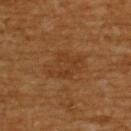Q: How large is the lesion?
A: ~5 mm (longest diameter)
Q: What kind of image is this?
A: 15 mm crop, total-body photography
Q: Lesion location?
A: the upper back
Q: Who is the patient?
A: male, about 60 years old
Q: What did automated image analysis measure?
A: an area of roughly 13 mm², a shape eccentricity near 0.75, and a shape-asymmetry score of about 0.25 (0 = symmetric); a lesion color around L≈35 a*≈19 b*≈32 in CIELAB, about 5 CIELAB-L* units darker than the surrounding skin, and a normalized lesion–skin contrast near 5; border irregularity of about 3.5 on a 0–10 scale, a within-lesion color-variation index near 2.5/10, and radial color variation of about 1; a classifier nevus-likeness of about 0/100 and lesion-presence confidence of about 100/100